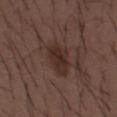Captured under white-light illumination.
On the mid back.
Automated image analysis of the tile measured a mean CIELAB color near L≈29 a*≈16 b*≈21 and a normalized border contrast of about 8. It also reported a border-irregularity rating of about 2.5/10 and a color-variation rating of about 3/10.
A close-up tile cropped from a whole-body skin photograph, about 15 mm across.
The patient is a male aged 48–52.